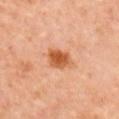Part of a total-body skin-imaging series; this lesion was reviewed on a skin check and was not flagged for biopsy.